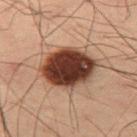* follow-up: imaged on a skin check; not biopsied
* patient: male, aged 53–57
* diameter: about 6.5 mm
* image: total-body-photography crop, ~15 mm field of view
* location: the left thigh